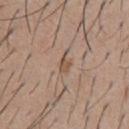workup: catalogued during a skin exam; not biopsied
illumination: white-light
patient: male, aged 58–62
image: total-body-photography crop, ~15 mm field of view
TBP lesion metrics: an area of roughly 3.5 mm² and an outline eccentricity of about 0.8 (0 = round, 1 = elongated); a border-irregularity rating of about 4/10 and radial color variation of about 1.5; an automated nevus-likeness rating near 0 out of 100 and lesion-presence confidence of about 100/100
location: the chest